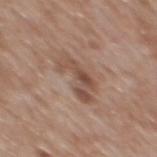location: the mid back
image source: ~15 mm crop, total-body skin-cancer survey
lighting: white-light
TBP lesion metrics: an average lesion color of about L≈50 a*≈18 b*≈26 (CIELAB), a lesion–skin lightness drop of about 9, and a normalized lesion–skin contrast near 7; a classifier nevus-likeness of about 10/100 and lesion-presence confidence of about 100/100
patient: male, aged around 60